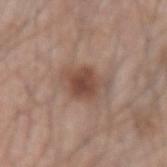A male subject aged approximately 45. A 15 mm close-up extracted from a 3D total-body photography capture. From the left forearm.